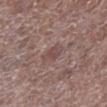The lesion was tiled from a total-body skin photograph and was not biopsied.